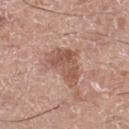Impression:
Captured during whole-body skin photography for melanoma surveillance; the lesion was not biopsied.
Background:
The lesion is located on the left thigh. The tile uses white-light illumination. A 15 mm crop from a total-body photograph taken for skin-cancer surveillance. A male patient, aged approximately 75. Measured at roughly 5 mm in maximum diameter. The total-body-photography lesion software estimated a border-irregularity index near 4.5/10, a within-lesion color-variation index near 3.5/10, and radial color variation of about 1.